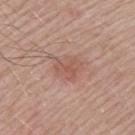The lesion was tiled from a total-body skin photograph and was not biopsied. Located on the left upper arm. This image is a 15 mm lesion crop taken from a total-body photograph. Measured at roughly 4 mm in maximum diameter. A male patient, roughly 65 years of age. Automated image analysis of the tile measured an outline eccentricity of about 0.7 (0 = round, 1 = elongated) and a symmetry-axis asymmetry near 0.3. The analysis additionally found a nevus-likeness score of about 50/100 and lesion-presence confidence of about 100/100. This is a white-light tile.